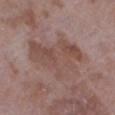- patient · female, approximately 70 years of age
- illumination · white-light illumination
- acquisition · 15 mm crop, total-body photography
- size · about 7.5 mm
- anatomic site · the left lower leg